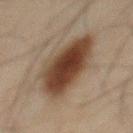Findings:
* follow-up: catalogued during a skin exam; not biopsied
* patient: male, about 45 years old
* site: the front of the torso
* lighting: cross-polarized illumination
* imaging modality: 15 mm crop, total-body photography
* size: ≈6.5 mm